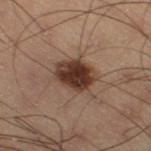Captured during whole-body skin photography for melanoma surveillance; the lesion was not biopsied. A male subject aged around 55. Cropped from a total-body skin-imaging series; the visible field is about 15 mm. On the right thigh.